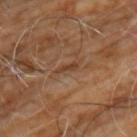Assessment: Part of a total-body skin-imaging series; this lesion was reviewed on a skin check and was not flagged for biopsy. Background: Imaged with cross-polarized lighting. From the front of the torso. A male subject aged approximately 60. The total-body-photography lesion software estimated a lesion-detection confidence of about 75/100. Measured at roughly 2.5 mm in maximum diameter. Cropped from a total-body skin-imaging series; the visible field is about 15 mm.